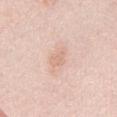No biopsy was performed on this lesion — it was imaged during a full skin examination and was not determined to be concerning. A roughly 15 mm field-of-view crop from a total-body skin photograph. A male subject approximately 25 years of age. Approximately 3 mm at its widest. Captured under white-light illumination. Located on the chest.